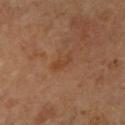This lesion was catalogued during total-body skin photography and was not selected for biopsy. From the right forearm. A 15 mm crop from a total-body photograph taken for skin-cancer surveillance. Measured at roughly 2.5 mm in maximum diameter. This is a cross-polarized tile. The patient is a female in their mid- to late 60s.